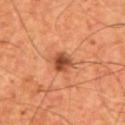Impression:
Captured during whole-body skin photography for melanoma surveillance; the lesion was not biopsied.
Clinical summary:
The tile uses cross-polarized illumination. The subject is a male in their mid-50s. Located on the front of the torso. Cropped from a whole-body photographic skin survey; the tile spans about 15 mm.